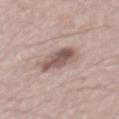biopsy status: no biopsy performed (imaged during a skin exam) | lighting: white-light illumination | TBP lesion metrics: an area of roughly 9.5 mm², a shape eccentricity near 0.9, and a symmetry-axis asymmetry near 0.25; internal color variation of about 5 on a 0–10 scale and a peripheral color-asymmetry measure near 1.5 | patient: male, about 55 years old | acquisition: ~15 mm crop, total-body skin-cancer survey.